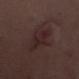Imaged during a routine full-body skin examination; the lesion was not biopsied and no histopathology is available.
A female subject, aged approximately 35.
Located on the abdomen.
The lesion's longest dimension is about 5 mm.
Cropped from a whole-body photographic skin survey; the tile spans about 15 mm.
This is a white-light tile.